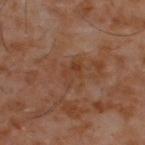No biopsy was performed on this lesion — it was imaged during a full skin examination and was not determined to be concerning.
The total-body-photography lesion software estimated a shape eccentricity near 0.8 and a symmetry-axis asymmetry near 0.4.
A male subject, aged 58–62.
On the upper back.
This is a cross-polarized tile.
Cropped from a whole-body photographic skin survey; the tile spans about 15 mm.
The recorded lesion diameter is about 3 mm.